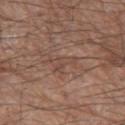image source: ~15 mm tile from a whole-body skin photo; tile lighting: white-light illumination; automated metrics: about 6 CIELAB-L* units darker than the surrounding skin and a normalized lesion–skin contrast near 4.5; body site: the left thigh; subject: male, in their 80s; diameter: ≈3.5 mm.